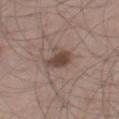No biopsy was performed on this lesion — it was imaged during a full skin examination and was not determined to be concerning. Automated tile analysis of the lesion measured a shape eccentricity near 0.7. A 15 mm close-up tile from a total-body photography series done for melanoma screening. The patient is a male aged 58–62. The lesion is located on the right thigh.